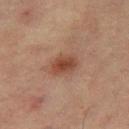Captured during whole-body skin photography for melanoma surveillance; the lesion was not biopsied.
This image is a 15 mm lesion crop taken from a total-body photograph.
Measured at roughly 3.5 mm in maximum diameter.
The lesion-visualizer software estimated an average lesion color of about L≈40 a*≈20 b*≈26 (CIELAB), about 9 CIELAB-L* units darker than the surrounding skin, and a normalized border contrast of about 8. And it measured a border-irregularity rating of about 1.5/10, a within-lesion color-variation index near 3.5/10, and a peripheral color-asymmetry measure near 1. It also reported a classifier nevus-likeness of about 90/100.
The lesion is located on the right thigh.
The patient is a female aged around 55.